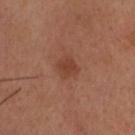| key | value |
|---|---|
| workup | catalogued during a skin exam; not biopsied |
| illumination | cross-polarized illumination |
| anatomic site | the head or neck |
| patient | male, about 65 years old |
| acquisition | ~15 mm crop, total-body skin-cancer survey |